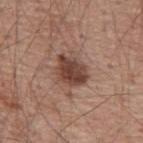biopsy_status: not biopsied; imaged during a skin examination
image:
  source: total-body photography crop
  field_of_view_mm: 15
site: mid back
patient:
  sex: male
  age_approx: 55
automated_metrics:
  eccentricity: 0.6
  shape_asymmetry: 0.2
  vs_skin_darker_L: 13.0
  lesion_detection_confidence_0_100: 100
lighting: white-light
lesion_size:
  long_diameter_mm_approx: 4.0